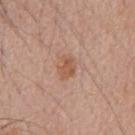Case summary:
- notes · no biopsy performed (imaged during a skin exam)
- subject · male, about 65 years old
- image · 15 mm crop, total-body photography
- location · the front of the torso